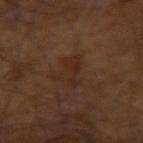notes = total-body-photography surveillance lesion; no biopsy
subject = male, approximately 60 years of age
body site = the arm
size = ~4 mm (longest diameter)
acquisition = total-body-photography crop, ~15 mm field of view
TBP lesion metrics = an area of roughly 6 mm² and an eccentricity of roughly 0.9; an average lesion color of about L≈25 a*≈17 b*≈24 (CIELAB), a lesion–skin lightness drop of about 4, and a normalized border contrast of about 5